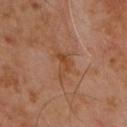<tbp_lesion>
  <biopsy_status>not biopsied; imaged during a skin examination</biopsy_status>
  <automated_metrics>
    <area_mm2_approx>6.0</area_mm2_approx>
    <eccentricity>0.85</eccentricity>
    <shape_asymmetry>0.6</shape_asymmetry>
    <cielab_L>42</cielab_L>
    <cielab_a>21</cielab_a>
    <cielab_b>31</cielab_b>
    <vs_skin_darker_L>6.0</vs_skin_darker_L>
    <vs_skin_contrast_norm>6.0</vs_skin_contrast_norm>
    <nevus_likeness_0_100>0</nevus_likeness_0_100>
    <lesion_detection_confidence_0_100>100</lesion_detection_confidence_0_100>
  </automated_metrics>
  <site>chest</site>
  <image>
    <source>total-body photography crop</source>
    <field_of_view_mm>15</field_of_view_mm>
  </image>
  <patient>
    <sex>male</sex>
    <age_approx>60</age_approx>
  </patient>
</tbp_lesion>